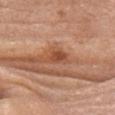Findings:
– follow-up · no biopsy performed (imaged during a skin exam)
– location · the head or neck
– illumination · white-light
– subject · female, in their mid- to late 70s
– image-analysis metrics · a mean CIELAB color near L≈49 a*≈26 b*≈34, about 9 CIELAB-L* units darker than the surrounding skin, and a normalized border contrast of about 7
– image source · total-body-photography crop, ~15 mm field of view